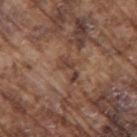Q: Is there a histopathology result?
A: total-body-photography surveillance lesion; no biopsy